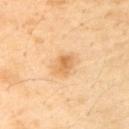The lesion was tiled from a total-body skin photograph and was not biopsied.